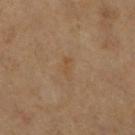Case summary:
– biopsy status — imaged on a skin check; not biopsied
– image source — 15 mm crop, total-body photography
– subject — female, roughly 55 years of age
– body site — the right lower leg